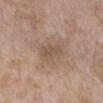notes: imaged on a skin check; not biopsied | image-analysis metrics: an area of roughly 4 mm², a shape eccentricity near 0.9, and a symmetry-axis asymmetry near 0.25; a mean CIELAB color near L≈52 a*≈17 b*≈26 and a normalized border contrast of about 5; a border-irregularity index near 3/10, a color-variation rating of about 1/10, and radial color variation of about 0.5; a classifier nevus-likeness of about 0/100 and lesion-presence confidence of about 100/100 | patient: female, roughly 55 years of age | size: ≈3 mm | anatomic site: the right lower leg | image source: ~15 mm crop, total-body skin-cancer survey | tile lighting: white-light.